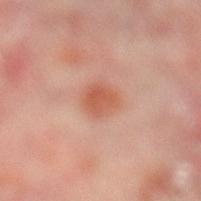Impression: Imaged during a routine full-body skin examination; the lesion was not biopsied and no histopathology is available. Acquisition and patient details: A male patient aged 58 to 62. Located on the left lower leg. A region of skin cropped from a whole-body photographic capture, roughly 15 mm wide. Imaged with cross-polarized lighting. The lesion's longest dimension is about 3 mm.